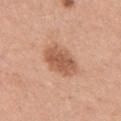{"biopsy_status": "not biopsied; imaged during a skin examination", "image": {"source": "total-body photography crop", "field_of_view_mm": 15}, "patient": {"sex": "female", "age_approx": 60}, "lesion_size": {"long_diameter_mm_approx": 4.5}, "lighting": "white-light", "site": "left upper arm"}